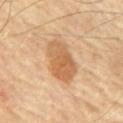This lesion was catalogued during total-body skin photography and was not selected for biopsy. The tile uses cross-polarized illumination. From the chest. The total-body-photography lesion software estimated an area of roughly 15 mm², an outline eccentricity of about 0.8 (0 = round, 1 = elongated), and a shape-asymmetry score of about 0.2 (0 = symmetric). The software also gave an average lesion color of about L≈60 a*≈20 b*≈38 (CIELAB), a lesion–skin lightness drop of about 11, and a lesion-to-skin contrast of about 7.5 (normalized; higher = more distinct). And it measured an automated nevus-likeness rating near 85 out of 100 and lesion-presence confidence of about 100/100. A male subject, aged 68–72. This image is a 15 mm lesion crop taken from a total-body photograph.